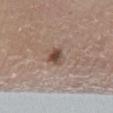| field | value |
|---|---|
| workup | catalogued during a skin exam; not biopsied |
| location | the right lower leg |
| illumination | white-light |
| TBP lesion metrics | an area of roughly 4 mm² and an eccentricity of roughly 0.65; a lesion color around L≈47 a*≈17 b*≈24 in CIELAB and roughly 12 lightness units darker than nearby skin; a border-irregularity rating of about 2.5/10, a color-variation rating of about 5/10, and peripheral color asymmetry of about 1.5 |
| diameter | about 2.5 mm |
| image source | total-body-photography crop, ~15 mm field of view |
| patient | male, aged approximately 65 |